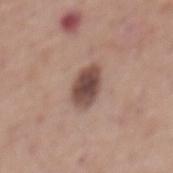Recorded during total-body skin imaging; not selected for excision or biopsy. The lesion's longest dimension is about 4 mm. Automated tile analysis of the lesion measured roughly 15 lightness units darker than nearby skin and a normalized lesion–skin contrast near 11. It also reported a within-lesion color-variation index near 4.5/10 and peripheral color asymmetry of about 1. And it measured an automated nevus-likeness rating near 90 out of 100. Located on the mid back. A roughly 15 mm field-of-view crop from a total-body skin photograph. A male subject, roughly 70 years of age.